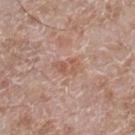workup — no biopsy performed (imaged during a skin exam)
location — the leg
acquisition — total-body-photography crop, ~15 mm field of view
patient — male, aged around 60
diameter — ~3 mm (longest diameter)
automated lesion analysis — an area of roughly 4 mm², a shape eccentricity near 0.8, and a shape-asymmetry score of about 0.35 (0 = symmetric); lesion-presence confidence of about 100/100